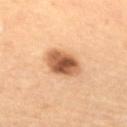The lesion was tiled from a total-body skin photograph and was not biopsied. The lesion is located on the right upper arm. An algorithmic analysis of the crop reported a lesion color around L≈54 a*≈22 b*≈35 in CIELAB and about 17 CIELAB-L* units darker than the surrounding skin. The software also gave a classifier nevus-likeness of about 90/100 and lesion-presence confidence of about 100/100. A female subject, aged 53–57. The recorded lesion diameter is about 4.5 mm. A 15 mm close-up extracted from a 3D total-body photography capture. This is a cross-polarized tile.